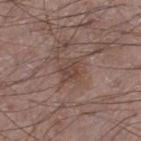Clinical impression: The lesion was tiled from a total-body skin photograph and was not biopsied. Image and clinical context: Measured at roughly 3 mm in maximum diameter. Located on the left thigh. Imaged with white-light lighting. A lesion tile, about 15 mm wide, cut from a 3D total-body photograph. A male subject, aged 48–52.